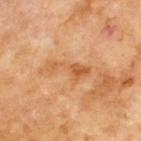Assessment: Recorded during total-body skin imaging; not selected for excision or biopsy. Clinical summary: Automated image analysis of the tile measured a shape eccentricity near 0.95 and two-axis asymmetry of about 0.6. The software also gave a detector confidence of about 100 out of 100 that the crop contains a lesion. The tile uses cross-polarized illumination. The lesion is on the upper back. The lesion's longest dimension is about 5 mm. A 15 mm close-up extracted from a 3D total-body photography capture. A male subject roughly 70 years of age.